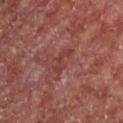| key | value |
|---|---|
| follow-up | total-body-photography surveillance lesion; no biopsy |
| imaging modality | ~15 mm crop, total-body skin-cancer survey |
| illumination | cross-polarized |
| automated metrics | a footprint of about 3 mm², an outline eccentricity of about 0.95 (0 = round, 1 = elongated), and a shape-asymmetry score of about 0.65 (0 = symmetric); lesion-presence confidence of about 70/100 |
| diameter | ≈3.5 mm |
| patient | male, aged 53 to 57 |
| location | the front of the torso |